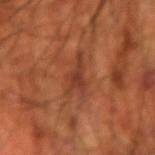<case>
<biopsy_status>not biopsied; imaged during a skin examination</biopsy_status>
<patient>
  <sex>male</sex>
  <age_approx>70</age_approx>
</patient>
<lesion_size>
  <long_diameter_mm_approx>5.0</long_diameter_mm_approx>
</lesion_size>
<site>left forearm</site>
<image>
  <source>total-body photography crop</source>
  <field_of_view_mm>15</field_of_view_mm>
</image>
<lighting>cross-polarized</lighting>
</case>